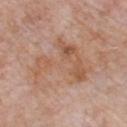Impression: No biopsy was performed on this lesion — it was imaged during a full skin examination and was not determined to be concerning. Image and clinical context: A male patient, in their 60s. Located on the chest. A region of skin cropped from a whole-body photographic capture, roughly 15 mm wide.